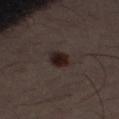Q: Was a biopsy performed?
A: imaged on a skin check; not biopsied
Q: Lesion location?
A: the front of the torso
Q: How was the tile lit?
A: cross-polarized illumination
Q: What is the lesion's diameter?
A: ≈2.5 mm
Q: How was this image acquired?
A: ~15 mm crop, total-body skin-cancer survey
Q: What are the patient's age and sex?
A: male, aged approximately 50